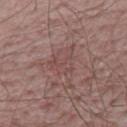• notes · no biopsy performed (imaged during a skin exam)
• imaging modality · ~15 mm crop, total-body skin-cancer survey
• size · ≈5.5 mm
• site · the mid back
• subject · male, about 65 years old
• illumination · white-light